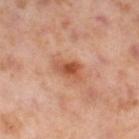<record>
  <patient>
    <sex>female</sex>
    <age_approx>55</age_approx>
  </patient>
  <image>
    <source>total-body photography crop</source>
    <field_of_view_mm>15</field_of_view_mm>
  </image>
  <site>right lower leg</site>
  <lighting>cross-polarized</lighting>
  <automated_metrics>
    <area_mm2_approx>6.0</area_mm2_approx>
    <eccentricity>0.8</eccentricity>
    <shape_asymmetry>0.3</shape_asymmetry>
    <border_irregularity_0_10>3.0</border_irregularity_0_10>
    <color_variation_0_10>4.5</color_variation_0_10>
    <peripheral_color_asymmetry>1.0</peripheral_color_asymmetry>
    <nevus_likeness_0_100>45</nevus_likeness_0_100>
    <lesion_detection_confidence_0_100>100</lesion_detection_confidence_0_100>
  </automated_metrics>
  <lesion_size>
    <long_diameter_mm_approx>3.5</long_diameter_mm_approx>
  </lesion_size>
</record>